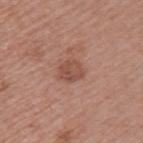Recorded during total-body skin imaging; not selected for excision or biopsy. The lesion is on the left upper arm. Captured under white-light illumination. A female subject, aged 43–47. Cropped from a whole-body photographic skin survey; the tile spans about 15 mm. About 3 mm across.